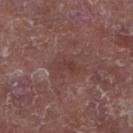workup = no biopsy performed (imaged during a skin exam) | image = ~15 mm tile from a whole-body skin photo | body site = the right lower leg | size = about 3 mm | illumination = white-light illumination | patient = male, aged around 65.